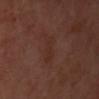Captured during whole-body skin photography for melanoma surveillance; the lesion was not biopsied. The lesion is on the upper back. A female subject, aged approximately 50. Approximately 3.5 mm at its widest. A 15 mm close-up extracted from a 3D total-body photography capture. The total-body-photography lesion software estimated a border-irregularity index near 4/10, a color-variation rating of about 1.5/10, and a peripheral color-asymmetry measure near 0.5. It also reported a nevus-likeness score of about 0/100 and a detector confidence of about 100 out of 100 that the crop contains a lesion.